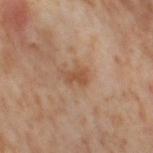workup: total-body-photography surveillance lesion; no biopsy
body site: the leg
diameter: ≈3 mm
imaging modality: total-body-photography crop, ~15 mm field of view
tile lighting: cross-polarized
patient: female, approximately 55 years of age
automated lesion analysis: peripheral color asymmetry of about 0.5; a nevus-likeness score of about 15/100 and lesion-presence confidence of about 100/100